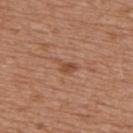The lesion was photographed on a routine skin check and not biopsied; there is no pathology result. Captured under white-light illumination. The patient is a male aged 63–67. Longest diameter approximately 3 mm. A lesion tile, about 15 mm wide, cut from a 3D total-body photograph. The lesion is on the back.